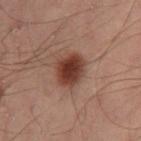Recorded during total-body skin imaging; not selected for excision or biopsy. Automated tile analysis of the lesion measured an average lesion color of about L≈31 a*≈19 b*≈22 (CIELAB), a lesion–skin lightness drop of about 12, and a normalized lesion–skin contrast near 11. And it measured border irregularity of about 1.5 on a 0–10 scale and internal color variation of about 4 on a 0–10 scale. The lesion is located on the left leg. The recorded lesion diameter is about 4 mm. Captured under cross-polarized illumination. A lesion tile, about 15 mm wide, cut from a 3D total-body photograph. The subject is a male aged 48 to 52.